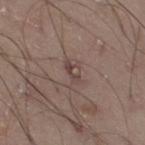Captured during whole-body skin photography for melanoma surveillance; the lesion was not biopsied. A close-up tile cropped from a whole-body skin photograph, about 15 mm across. This is a white-light tile. Measured at roughly 2.5 mm in maximum diameter. The lesion-visualizer software estimated a footprint of about 2.5 mm² and two-axis asymmetry of about 0.35. On the right thigh. A male patient, aged 18–22.